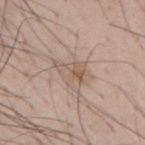Impression:
No biopsy was performed on this lesion — it was imaged during a full skin examination and was not determined to be concerning.
Background:
A male subject in their mid- to late 50s. This is a white-light tile. The lesion-visualizer software estimated internal color variation of about 6.5 on a 0–10 scale and a peripheral color-asymmetry measure near 2.5. It also reported a nevus-likeness score of about 0/100 and a detector confidence of about 100 out of 100 that the crop contains a lesion. A 15 mm close-up tile from a total-body photography series done for melanoma screening. The lesion is on the mid back.